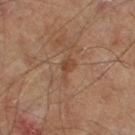location: the right leg | acquisition: total-body-photography crop, ~15 mm field of view | patient: male, aged 58 to 62 | lesion size: ≈2.5 mm.